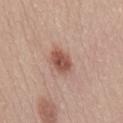The lesion was photographed on a routine skin check and not biopsied; there is no pathology result. On the back. A female subject, aged 48 to 52. A 15 mm close-up extracted from a 3D total-body photography capture.